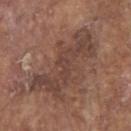No biopsy was performed on this lesion — it was imaged during a full skin examination and was not determined to be concerning. The lesion is located on the head or neck. Measured at roughly 8.5 mm in maximum diameter. The total-body-photography lesion software estimated a lesion area of about 22 mm² and an outline eccentricity of about 0.9 (0 = round, 1 = elongated). It also reported an average lesion color of about L≈42 a*≈18 b*≈23 (CIELAB) and a lesion–skin lightness drop of about 8. It also reported a border-irregularity index near 8.5/10, internal color variation of about 3 on a 0–10 scale, and radial color variation of about 1. And it measured a classifier nevus-likeness of about 0/100 and lesion-presence confidence of about 80/100. The subject is a male aged around 80. This image is a 15 mm lesion crop taken from a total-body photograph.